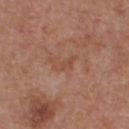Part of a total-body skin-imaging series; this lesion was reviewed on a skin check and was not flagged for biopsy. The patient is a male roughly 55 years of age. A lesion tile, about 15 mm wide, cut from a 3D total-body photograph. Located on the chest. Automated image analysis of the tile measured a border-irregularity index near 5/10, a within-lesion color-variation index near 0/10, and radial color variation of about 0. Imaged with white-light lighting.